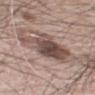workup: total-body-photography surveillance lesion; no biopsy
image-analysis metrics: a classifier nevus-likeness of about 90/100 and a lesion-detection confidence of about 100/100
patient: male, aged 58–62
body site: the mid back
acquisition: ~15 mm tile from a whole-body skin photo
size: ≈7 mm
illumination: white-light